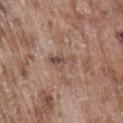Notes:
* biopsy status — imaged on a skin check; not biopsied
* subject — male, aged 73 to 77
* body site — the back
* acquisition — 15 mm crop, total-body photography